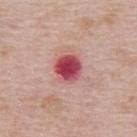image = 15 mm crop, total-body photography; site = the upper back; subject = female, aged around 65; automated lesion analysis = an automated nevus-likeness rating near 0 out of 100 and a detector confidence of about 100 out of 100 that the crop contains a lesion; illumination = white-light illumination; size = about 3.5 mm.